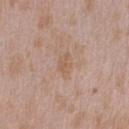Q: Was this lesion biopsied?
A: no biopsy performed (imaged during a skin exam)
Q: What kind of image is this?
A: 15 mm crop, total-body photography
Q: Lesion size?
A: about 3 mm
Q: Where on the body is the lesion?
A: the right upper arm
Q: Illumination type?
A: white-light
Q: Who is the patient?
A: male, in their mid-40s